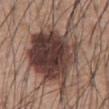- notes · total-body-photography surveillance lesion; no biopsy
- acquisition · total-body-photography crop, ~15 mm field of view
- body site · the abdomen
- subject · male, about 60 years old
- size · ~12 mm (longest diameter)
- illumination · white-light
- image-analysis metrics · an area of roughly 50 mm² and two-axis asymmetry of about 0.3; a classifier nevus-likeness of about 75/100